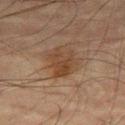{
  "biopsy_status": "not biopsied; imaged during a skin examination",
  "lighting": "cross-polarized",
  "lesion_size": {
    "long_diameter_mm_approx": 4.0
  },
  "site": "leg",
  "image": {
    "source": "total-body photography crop",
    "field_of_view_mm": 15
  },
  "patient": {
    "sex": "male",
    "age_approx": 75
  }
}